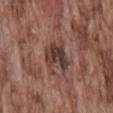biopsy_status: not biopsied; imaged during a skin examination
site: mid back
patient:
  sex: male
  age_approx: 75
lesion_size:
  long_diameter_mm_approx: 4.0
automated_metrics:
  area_mm2_approx: 8.5
  eccentricity: 0.75
  shape_asymmetry: 0.35
lighting: white-light
image:
  source: total-body photography crop
  field_of_view_mm: 15